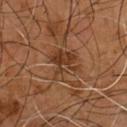Clinical summary: A close-up tile cropped from a whole-body skin photograph, about 15 mm across. An algorithmic analysis of the crop reported an area of roughly 10 mm² and a shape eccentricity near 0.55. The analysis additionally found an average lesion color of about L≈34 a*≈19 b*≈29 (CIELAB), about 8 CIELAB-L* units darker than the surrounding skin, and a normalized border contrast of about 7. And it measured a border-irregularity index near 6.5/10, internal color variation of about 5 on a 0–10 scale, and peripheral color asymmetry of about 1.5. The recorded lesion diameter is about 4 mm. This is a cross-polarized tile. A male patient aged approximately 55. From the front of the torso.